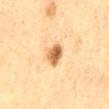automated lesion analysis: a lesion area of about 5 mm², an outline eccentricity of about 0.55 (0 = round, 1 = elongated), and a symmetry-axis asymmetry near 0.2; about 15 CIELAB-L* units darker than the surrounding skin and a lesion-to-skin contrast of about 10 (normalized; higher = more distinct); an automated nevus-likeness rating near 100 out of 100 | body site: the chest | size: about 3 mm | imaging modality: ~15 mm crop, total-body skin-cancer survey | subject: female, approximately 55 years of age.